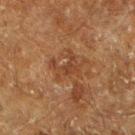This lesion was catalogued during total-body skin photography and was not selected for biopsy. On the left lower leg. The patient is a male in their 60s. A lesion tile, about 15 mm wide, cut from a 3D total-body photograph. An algorithmic analysis of the crop reported a mean CIELAB color near L≈32 a*≈18 b*≈27, about 6 CIELAB-L* units darker than the surrounding skin, and a normalized lesion–skin contrast near 5.5. The analysis additionally found a nevus-likeness score of about 0/100 and a lesion-detection confidence of about 95/100.